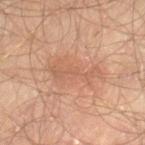The lesion was tiled from a total-body skin photograph and was not biopsied.
Automated image analysis of the tile measured border irregularity of about 7.5 on a 0–10 scale and internal color variation of about 2 on a 0–10 scale.
The tile uses cross-polarized illumination.
The lesion's longest dimension is about 6.5 mm.
A close-up tile cropped from a whole-body skin photograph, about 15 mm across.
The subject is a male aged around 65.
On the left thigh.